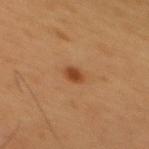Clinical impression:
Recorded during total-body skin imaging; not selected for excision or biopsy.
Acquisition and patient details:
Located on the mid back. The recorded lesion diameter is about 2.5 mm. This is a cross-polarized tile. A male patient, in their mid-50s. A region of skin cropped from a whole-body photographic capture, roughly 15 mm wide. An algorithmic analysis of the crop reported an average lesion color of about L≈39 a*≈22 b*≈34 (CIELAB) and a lesion-to-skin contrast of about 8.5 (normalized; higher = more distinct). The analysis additionally found a classifier nevus-likeness of about 95/100.